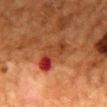Assessment:
No biopsy was performed on this lesion — it was imaged during a full skin examination and was not determined to be concerning.
Acquisition and patient details:
About 5.5 mm across. From the mid back. A female subject in their 50s. Cropped from a whole-body photographic skin survey; the tile spans about 15 mm. The total-body-photography lesion software estimated an area of roughly 10 mm², an eccentricity of roughly 0.9, and a shape-asymmetry score of about 0.45 (0 = symmetric). It also reported a mean CIELAB color near L≈34 a*≈27 b*≈31, a lesion–skin lightness drop of about 9, and a normalized lesion–skin contrast near 7.5. The software also gave a nevus-likeness score of about 0/100.